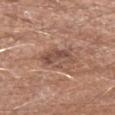Notes:
• biopsy status · imaged on a skin check; not biopsied
• lighting · white-light
• lesion size · about 3.5 mm
• anatomic site · the left forearm
• image source · total-body-photography crop, ~15 mm field of view
• subject · male, aged approximately 65